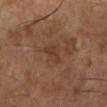The lesion was tiled from a total-body skin photograph and was not biopsied.